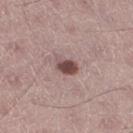The lesion was tiled from a total-body skin photograph and was not biopsied.
A male patient aged 43 to 47.
The lesion is located on the right thigh.
A lesion tile, about 15 mm wide, cut from a 3D total-body photograph.
An algorithmic analysis of the crop reported a border-irregularity index near 2.5/10 and peripheral color asymmetry of about 0.5.
Measured at roughly 3 mm in maximum diameter.